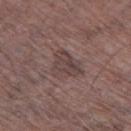Recorded during total-body skin imaging; not selected for excision or biopsy.
A region of skin cropped from a whole-body photographic capture, roughly 15 mm wide.
A male subject, aged around 75.
An algorithmic analysis of the crop reported a border-irregularity index near 3.5/10, a within-lesion color-variation index near 3.5/10, and a peripheral color-asymmetry measure near 1.5. And it measured a classifier nevus-likeness of about 0/100 and lesion-presence confidence of about 85/100.
About 4 mm across.
Located on the leg.
This is a white-light tile.